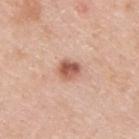Part of a total-body skin-imaging series; this lesion was reviewed on a skin check and was not flagged for biopsy.
A male patient, approximately 45 years of age.
A 15 mm close-up extracted from a 3D total-body photography capture.
Located on the upper back.
An algorithmic analysis of the crop reported a footprint of about 5 mm², a shape eccentricity near 0.55, and a shape-asymmetry score of about 0.15 (0 = symmetric).